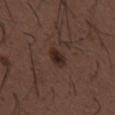The lesion was photographed on a routine skin check and not biopsied; there is no pathology result. Imaged with white-light lighting. A male patient, aged 48–52. On the front of the torso. Cropped from a total-body skin-imaging series; the visible field is about 15 mm.